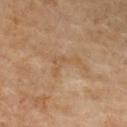biopsy status: total-body-photography surveillance lesion; no biopsy | imaging modality: 15 mm crop, total-body photography | subject: female, about 50 years old | lighting: cross-polarized | location: the chest | lesion size: ≈4 mm.